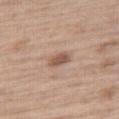No biopsy was performed on this lesion — it was imaged during a full skin examination and was not determined to be concerning.
Imaged with white-light lighting.
A 15 mm close-up extracted from a 3D total-body photography capture.
A male subject, about 70 years old.
Automated tile analysis of the lesion measured an automated nevus-likeness rating near 50 out of 100 and lesion-presence confidence of about 100/100.
Located on the right thigh.